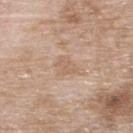Q: Was this lesion biopsied?
A: catalogued during a skin exam; not biopsied
Q: Lesion location?
A: the upper back
Q: What did automated image analysis measure?
A: an average lesion color of about L≈61 a*≈17 b*≈30 (CIELAB), roughly 6 lightness units darker than nearby skin, and a normalized lesion–skin contrast near 4.5; a classifier nevus-likeness of about 0/100 and a detector confidence of about 100 out of 100 that the crop contains a lesion
Q: What is the lesion's diameter?
A: ~2.5 mm (longest diameter)
Q: What kind of image is this?
A: total-body-photography crop, ~15 mm field of view
Q: What are the patient's age and sex?
A: female, aged 73 to 77
Q: How was the tile lit?
A: white-light